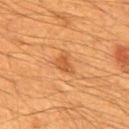Q: Is there a histopathology result?
A: total-body-photography surveillance lesion; no biopsy
Q: How was the tile lit?
A: cross-polarized
Q: Lesion size?
A: ~2.5 mm (longest diameter)
Q: Who is the patient?
A: male, roughly 60 years of age
Q: Lesion location?
A: the mid back
Q: What is the imaging modality?
A: 15 mm crop, total-body photography
Q: What did automated image analysis measure?
A: a mean CIELAB color near L≈52 a*≈26 b*≈42, a lesion–skin lightness drop of about 9, and a normalized border contrast of about 6; border irregularity of about 3 on a 0–10 scale, a color-variation rating of about 2/10, and a peripheral color-asymmetry measure near 0.5